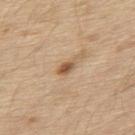<tbp_lesion>
<biopsy_status>not biopsied; imaged during a skin examination</biopsy_status>
<site>upper back</site>
<lighting>white-light</lighting>
<patient>
  <sex>male</sex>
  <age_approx>70</age_approx>
</patient>
<automated_metrics>
  <area_mm2_approx>3.5</area_mm2_approx>
  <eccentricity>0.85</eccentricity>
  <shape_asymmetry>0.2</shape_asymmetry>
  <cielab_L>56</cielab_L>
  <cielab_a>18</cielab_a>
  <cielab_b>34</cielab_b>
  <vs_skin_darker_L>12.0</vs_skin_darker_L>
  <vs_skin_contrast_norm>8.0</vs_skin_contrast_norm>
  <nevus_likeness_0_100>60</nevus_likeness_0_100>
  <lesion_detection_confidence_0_100>100</lesion_detection_confidence_0_100>
</automated_metrics>
<image>
  <source>total-body photography crop</source>
  <field_of_view_mm>15</field_of_view_mm>
</image>
<lesion_size>
  <long_diameter_mm_approx>2.5</long_diameter_mm_approx>
</lesion_size>
</tbp_lesion>